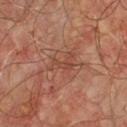biopsy_status: not biopsied; imaged during a skin examination
site: chest
automated_metrics:
  area_mm2_approx: 5.0
  eccentricity: 0.85
  border_irregularity_0_10: 7.0
  color_variation_0_10: 2.0
  peripheral_color_asymmetry: 0.5
  nevus_likeness_0_100: 0
  lesion_detection_confidence_0_100: 100
image:
  source: total-body photography crop
  field_of_view_mm: 15
patient:
  sex: male
  age_approx: 55
lighting: cross-polarized
lesion_size:
  long_diameter_mm_approx: 4.0